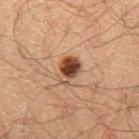{"biopsy_status": "not biopsied; imaged during a skin examination", "automated_metrics": {"eccentricity": 0.75, "shape_asymmetry": 0.3, "cielab_L": 37, "cielab_a": 19, "cielab_b": 27, "vs_skin_darker_L": 15.0, "vs_skin_contrast_norm": 12.0}, "site": "right thigh", "lesion_size": {"long_diameter_mm_approx": 3.0}, "lighting": "cross-polarized", "image": {"source": "total-body photography crop", "field_of_view_mm": 15}, "patient": {"sex": "male", "age_approx": 65}}